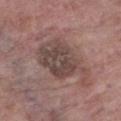Clinical impression:
The lesion was photographed on a routine skin check and not biopsied; there is no pathology result.
Background:
Longest diameter approximately 7 mm. The tile uses white-light illumination. A female subject aged approximately 85 or older. From the left lower leg. A lesion tile, about 15 mm wide, cut from a 3D total-body photograph.